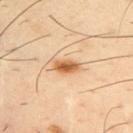Clinical impression: Recorded during total-body skin imaging; not selected for excision or biopsy. Background: The lesion is on the right upper arm. A 15 mm close-up extracted from a 3D total-body photography capture. A male patient about 40 years old.